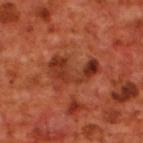Cropped from a total-body skin-imaging series; the visible field is about 15 mm. On the upper back. The patient is a male aged 68 to 72. The tile uses cross-polarized illumination. An algorithmic analysis of the crop reported an outline eccentricity of about 0.85 (0 = round, 1 = elongated) and two-axis asymmetry of about 0.55. The analysis additionally found an automated nevus-likeness rating near 0 out of 100 and a lesion-detection confidence of about 100/100. About 5.5 mm across.